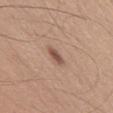Imaged during a routine full-body skin examination; the lesion was not biopsied and no histopathology is available. The lesion is located on the front of the torso. Imaged with white-light lighting. A 15 mm crop from a total-body photograph taken for skin-cancer surveillance. Automated tile analysis of the lesion measured a footprint of about 3.5 mm², a shape eccentricity near 0.85, and a shape-asymmetry score of about 0.2 (0 = symmetric). It also reported a lesion color around L≈52 a*≈20 b*≈26 in CIELAB and roughly 11 lightness units darker than nearby skin. The software also gave a border-irregularity index near 2.5/10, a color-variation rating of about 2.5/10, and a peripheral color-asymmetry measure near 1. And it measured a classifier nevus-likeness of about 90/100 and a detector confidence of about 100 out of 100 that the crop contains a lesion. The lesion's longest dimension is about 2.5 mm. A male subject, in their mid-60s.